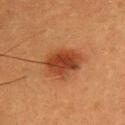Impression:
No biopsy was performed on this lesion — it was imaged during a full skin examination and was not determined to be concerning.
Background:
A male patient in their mid- to late 50s. This is a cross-polarized tile. Cropped from a total-body skin-imaging series; the visible field is about 15 mm. Measured at roughly 5 mm in maximum diameter. An algorithmic analysis of the crop reported a within-lesion color-variation index near 4.5/10. The software also gave a classifier nevus-likeness of about 100/100 and a detector confidence of about 100 out of 100 that the crop contains a lesion. The lesion is on the upper back.